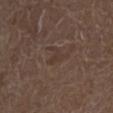This lesion was catalogued during total-body skin photography and was not selected for biopsy.
Automated image analysis of the tile measured a mean CIELAB color near L≈35 a*≈13 b*≈22 and roughly 4 lightness units darker than nearby skin. The software also gave a detector confidence of about 100 out of 100 that the crop contains a lesion.
This is a white-light tile.
The recorded lesion diameter is about 2.5 mm.
A roughly 15 mm field-of-view crop from a total-body skin photograph.
On the left lower leg.
A male subject, about 75 years old.